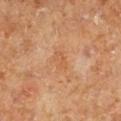workup = catalogued during a skin exam; not biopsied | body site = the left lower leg | patient = female | illumination = cross-polarized | image source = ~15 mm tile from a whole-body skin photo.